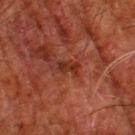No biopsy was performed on this lesion — it was imaged during a full skin examination and was not determined to be concerning.
A male subject aged approximately 80.
On the left thigh.
A close-up tile cropped from a whole-body skin photograph, about 15 mm across.